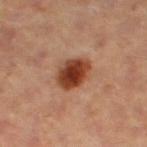Part of a total-body skin-imaging series; this lesion was reviewed on a skin check and was not flagged for biopsy. A roughly 15 mm field-of-view crop from a total-body skin photograph. Measured at roughly 4 mm in maximum diameter. An algorithmic analysis of the crop reported a lesion–skin lightness drop of about 16 and a normalized lesion–skin contrast near 12.5. Captured under cross-polarized illumination. On the right thigh. A female subject, aged 38 to 42.